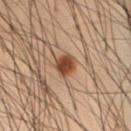Q: Was a biopsy performed?
A: catalogued during a skin exam; not biopsied
Q: Automated lesion metrics?
A: border irregularity of about 1.5 on a 0–10 scale, a color-variation rating of about 2/10, and a peripheral color-asymmetry measure near 0.5
Q: What is the lesion's diameter?
A: ≈2.5 mm
Q: Illumination type?
A: cross-polarized illumination
Q: Patient demographics?
A: male, about 55 years old
Q: How was this image acquired?
A: total-body-photography crop, ~15 mm field of view
Q: What is the anatomic site?
A: the front of the torso